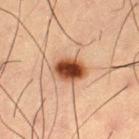  biopsy_status: not biopsied; imaged during a skin examination
  site: right thigh
  patient:
    sex: male
    age_approx: 55
  image:
    source: total-body photography crop
    field_of_view_mm: 15